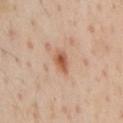This lesion was catalogued during total-body skin photography and was not selected for biopsy.
The lesion is located on the chest.
This image is a 15 mm lesion crop taken from a total-body photograph.
A male subject, about 55 years old.
About 3 mm across.
The tile uses cross-polarized illumination.